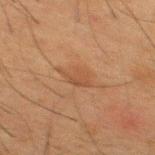Findings:
– follow-up · no biopsy performed (imaged during a skin exam)
– body site · the mid back
– patient · male, about 35 years old
– lesion size · about 3.5 mm
– imaging modality · ~15 mm crop, total-body skin-cancer survey